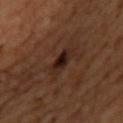• imaging modality — ~15 mm tile from a whole-body skin photo
• TBP lesion metrics — a lesion area of about 6 mm², an eccentricity of roughly 0.9, and two-axis asymmetry of about 0.35; border irregularity of about 3.5 on a 0–10 scale
• tile lighting — cross-polarized
• anatomic site — the upper back
• size — about 4 mm
• patient — male, aged around 50
• biopsy diagnosis — an atypical melanocytic neoplasm (borderline)